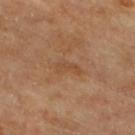Q: Is there a histopathology result?
A: total-body-photography surveillance lesion; no biopsy